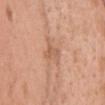{"biopsy_status": "not biopsied; imaged during a skin examination", "image": {"source": "total-body photography crop", "field_of_view_mm": 15}, "site": "chest", "lighting": "white-light", "patient": {"sex": "female", "age_approx": 50}}